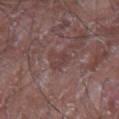Q: Was this lesion biopsied?
A: total-body-photography surveillance lesion; no biopsy
Q: Where on the body is the lesion?
A: the right upper arm
Q: What did automated image analysis measure?
A: a lesion area of about 4.5 mm² and an outline eccentricity of about 0.65 (0 = round, 1 = elongated); a lesion–skin lightness drop of about 6; a peripheral color-asymmetry measure near 0.5
Q: What are the patient's age and sex?
A: male, aged 63 to 67
Q: How large is the lesion?
A: ≈3 mm
Q: How was the tile lit?
A: white-light
Q: What kind of image is this?
A: total-body-photography crop, ~15 mm field of view